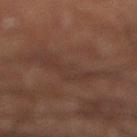{"biopsy_status": "not biopsied; imaged during a skin examination", "site": "leg", "lesion_size": {"long_diameter_mm_approx": 7.5}, "patient": {"sex": "male", "age_approx": 60}, "image": {"source": "total-body photography crop", "field_of_view_mm": 15}}